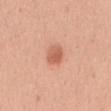Notes:
• biopsy status — total-body-photography surveillance lesion; no biopsy
• automated metrics — a footprint of about 4.5 mm²
• location — the mid back
• lesion diameter — about 2.5 mm
• tile lighting — white-light illumination
• subject — female, approximately 55 years of age
• imaging modality — total-body-photography crop, ~15 mm field of view